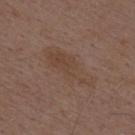Findings:
• workup · imaged on a skin check; not biopsied
• automated lesion analysis · an area of roughly 14 mm² and a symmetry-axis asymmetry near 0.25; a mean CIELAB color near L≈43 a*≈16 b*≈25, about 5 CIELAB-L* units darker than the surrounding skin, and a normalized border contrast of about 5; a border-irregularity rating of about 4.5/10, internal color variation of about 2.5 on a 0–10 scale, and a peripheral color-asymmetry measure near 1
• image source · ~15 mm tile from a whole-body skin photo
• tile lighting · white-light illumination
• subject · male, in their 50s
• diameter · about 6.5 mm
• anatomic site · the mid back